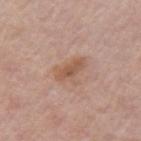Impression:
This lesion was catalogued during total-body skin photography and was not selected for biopsy.
Clinical summary:
On the left upper arm. This is a white-light tile. Longest diameter approximately 3.5 mm. A 15 mm close-up tile from a total-body photography series done for melanoma screening. A female subject aged 58 to 62.